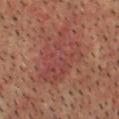Impression: Imaged during a routine full-body skin examination; the lesion was not biopsied and no histopathology is available. Acquisition and patient details: This is a cross-polarized tile. Cropped from a total-body skin-imaging series; the visible field is about 15 mm. The lesion is located on the head or neck. The recorded lesion diameter is about 7.5 mm. A male subject, approximately 60 years of age.